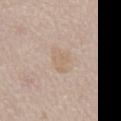The lesion's longest dimension is about 4 mm.
The total-body-photography lesion software estimated a lesion area of about 5 mm² and a shape-asymmetry score of about 0.35 (0 = symmetric). The software also gave a lesion color around L≈64 a*≈13 b*≈29 in CIELAB, a lesion–skin lightness drop of about 5, and a normalized lesion–skin contrast near 4.5. It also reported border irregularity of about 4 on a 0–10 scale, a within-lesion color-variation index near 1.5/10, and radial color variation of about 0.5. And it measured lesion-presence confidence of about 100/100.
This image is a 15 mm lesion crop taken from a total-body photograph.
The subject is a male aged 63–67.
The lesion is located on the front of the torso.